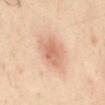site — the back | lighting — cross-polarized illumination | subject — male, aged approximately 40 | imaging modality — 15 mm crop, total-body photography | diameter — ≈4 mm.